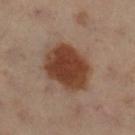Background: An algorithmic analysis of the crop reported an automated nevus-likeness rating near 100 out of 100. The tile uses cross-polarized illumination. The lesion is on the left leg. The patient is a female approximately 60 years of age. This image is a 15 mm lesion crop taken from a total-body photograph. The recorded lesion diameter is about 6 mm.